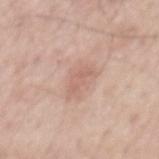Image and clinical context: A close-up tile cropped from a whole-body skin photograph, about 15 mm across. About 3.5 mm across. Captured under white-light illumination. The subject is a male aged approximately 60. The lesion-visualizer software estimated a lesion color around L≈62 a*≈21 b*≈26 in CIELAB. From the mid back.